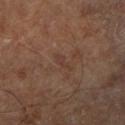{
  "image": {
    "source": "total-body photography crop",
    "field_of_view_mm": 15
  },
  "lesion_size": {
    "long_diameter_mm_approx": 3.0
  },
  "site": "leg",
  "patient": {
    "age_approx": 65
  }
}